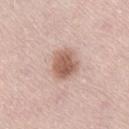Assessment:
Recorded during total-body skin imaging; not selected for excision or biopsy.
Acquisition and patient details:
A female patient aged 63 to 67. The lesion is on the lower back. This is a white-light tile. About 4 mm across. A 15 mm close-up extracted from a 3D total-body photography capture.